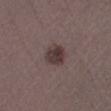{"biopsy_status": "not biopsied; imaged during a skin examination", "site": "left lower leg", "image": {"source": "total-body photography crop", "field_of_view_mm": 15}, "patient": {"sex": "male", "age_approx": 40}}